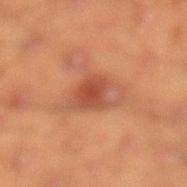Findings:
- biopsy status — imaged on a skin check; not biopsied
- anatomic site — the left lower leg
- automated lesion analysis — a mean CIELAB color near L≈43 a*≈23 b*≈29, about 8 CIELAB-L* units darker than the surrounding skin, and a lesion-to-skin contrast of about 6.5 (normalized; higher = more distinct); a classifier nevus-likeness of about 65/100 and a lesion-detection confidence of about 100/100
- size — about 6 mm
- illumination — cross-polarized
- patient — male, aged 43 to 47
- imaging modality — ~15 mm tile from a whole-body skin photo